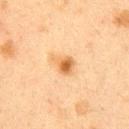Impression: Part of a total-body skin-imaging series; this lesion was reviewed on a skin check and was not flagged for biopsy. Image and clinical context: Imaged with cross-polarized lighting. A 15 mm crop from a total-body photograph taken for skin-cancer surveillance. The subject is a female in their 40s. On the right upper arm.